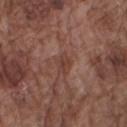workup: imaged on a skin check; not biopsied | illumination: white-light | acquisition: 15 mm crop, total-body photography | lesion diameter: ≈2.5 mm | patient: male, in their mid- to late 70s | location: the right upper arm.